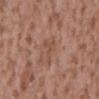Assessment: Recorded during total-body skin imaging; not selected for excision or biopsy. Background: A male patient about 45 years old. A region of skin cropped from a whole-body photographic capture, roughly 15 mm wide. Longest diameter approximately 3 mm. The total-body-photography lesion software estimated a lesion area of about 3.5 mm² and a symmetry-axis asymmetry near 0.65. The software also gave a border-irregularity rating of about 7.5/10, internal color variation of about 0 on a 0–10 scale, and a peripheral color-asymmetry measure near 0. The software also gave an automated nevus-likeness rating near 0 out of 100 and a detector confidence of about 100 out of 100 that the crop contains a lesion. Imaged with white-light lighting. Located on the mid back.